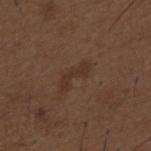| feature | finding |
|---|---|
| notes | no biopsy performed (imaged during a skin exam) |
| location | the upper back |
| subject | male, approximately 50 years of age |
| automated lesion analysis | a footprint of about 3.5 mm², an eccentricity of roughly 0.95, and a symmetry-axis asymmetry near 0.55; a mean CIELAB color near L≈31 a*≈17 b*≈24, roughly 6 lightness units darker than nearby skin, and a lesion-to-skin contrast of about 5.5 (normalized; higher = more distinct); a within-lesion color-variation index near 0/10 |
| image source | ~15 mm crop, total-body skin-cancer survey |